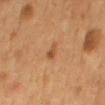Imaged during a routine full-body skin examination; the lesion was not biopsied and no histopathology is available.
Located on the mid back.
The tile uses cross-polarized illumination.
A male subject approximately 55 years of age.
A region of skin cropped from a whole-body photographic capture, roughly 15 mm wide.
The recorded lesion diameter is about 2.5 mm.
The total-body-photography lesion software estimated border irregularity of about 3 on a 0–10 scale, internal color variation of about 1.5 on a 0–10 scale, and a peripheral color-asymmetry measure near 0.5.